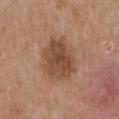A 15 mm close-up extracted from a 3D total-body photography capture. About 6 mm across. From the right upper arm. A male patient, aged around 50.